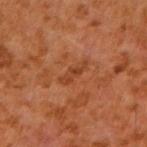Captured during whole-body skin photography for melanoma surveillance; the lesion was not biopsied.
Imaged with cross-polarized lighting.
A 15 mm close-up extracted from a 3D total-body photography capture.
Measured at roughly 3.5 mm in maximum diameter.
The subject is a male aged approximately 60.
The lesion is on the right upper arm.
An algorithmic analysis of the crop reported a lesion area of about 3 mm² and a symmetry-axis asymmetry near 0.45. The software also gave a border-irregularity rating of about 5.5/10, internal color variation of about 0 on a 0–10 scale, and a peripheral color-asymmetry measure near 0.